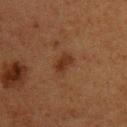notes: imaged on a skin check; not biopsied | image: ~15 mm tile from a whole-body skin photo | illumination: cross-polarized illumination | automated metrics: an outline eccentricity of about 0.8 (0 = round, 1 = elongated) and two-axis asymmetry of about 0.25; border irregularity of about 2.5 on a 0–10 scale, a within-lesion color-variation index near 1.5/10, and radial color variation of about 0.5 | site: the upper back | patient: female, roughly 40 years of age | size: ≈3 mm.